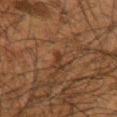Captured during whole-body skin photography for melanoma surveillance; the lesion was not biopsied.
Measured at roughly 1.5 mm in maximum diameter.
From the arm.
The tile uses cross-polarized illumination.
A male patient, in their mid-60s.
A region of skin cropped from a whole-body photographic capture, roughly 15 mm wide.